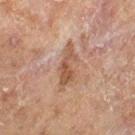Imaged during a routine full-body skin examination; the lesion was not biopsied and no histopathology is available. A 15 mm close-up extracted from a 3D total-body photography capture. Longest diameter approximately 5.5 mm. The patient is a male aged 68–72. The lesion is on the left thigh.